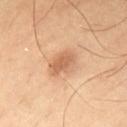Imaged during a routine full-body skin examination; the lesion was not biopsied and no histopathology is available.
A male subject approximately 40 years of age.
About 3.5 mm across.
A lesion tile, about 15 mm wide, cut from a 3D total-body photograph.
Automated tile analysis of the lesion measured a shape-asymmetry score of about 0.2 (0 = symmetric). And it measured a mean CIELAB color near L≈61 a*≈21 b*≈35 and about 10 CIELAB-L* units darker than the surrounding skin. The software also gave a peripheral color-asymmetry measure near 1. It also reported a nevus-likeness score of about 60/100.
Captured under cross-polarized illumination.
On the chest.